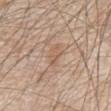The lesion was photographed on a routine skin check and not biopsied; there is no pathology result. A male subject aged 78–82. Measured at roughly 3.5 mm in maximum diameter. This is a white-light tile. The lesion is on the chest. A 15 mm close-up tile from a total-body photography series done for melanoma screening.